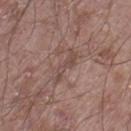Part of a total-body skin-imaging series; this lesion was reviewed on a skin check and was not flagged for biopsy.
The lesion-visualizer software estimated a lesion color around L≈47 a*≈18 b*≈22 in CIELAB and a normalized lesion–skin contrast near 5.5. The software also gave a nevus-likeness score of about 0/100 and a detector confidence of about 55 out of 100 that the crop contains a lesion.
The subject is a male in their mid-50s.
This is a white-light tile.
Longest diameter approximately 4 mm.
A 15 mm crop from a total-body photograph taken for skin-cancer surveillance.
From the left thigh.